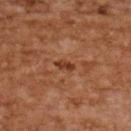Clinical impression: Part of a total-body skin-imaging series; this lesion was reviewed on a skin check and was not flagged for biopsy. Clinical summary: From the upper back. Longest diameter approximately 2.5 mm. Captured under cross-polarized illumination. The subject is a female roughly 60 years of age. This image is a 15 mm lesion crop taken from a total-body photograph.